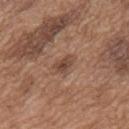Recorded during total-body skin imaging; not selected for excision or biopsy.
The patient is a male aged 73 to 77.
Cropped from a total-body skin-imaging series; the visible field is about 15 mm.
From the upper back.
The tile uses white-light illumination.